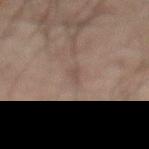Imaged during a routine full-body skin examination; the lesion was not biopsied and no histopathology is available.
The lesion is on the abdomen.
Measured at roughly 2.5 mm in maximum diameter.
A region of skin cropped from a whole-body photographic capture, roughly 15 mm wide.
A male subject approximately 70 years of age.
Captured under cross-polarized illumination.